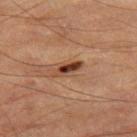Impression: Recorded during total-body skin imaging; not selected for excision or biopsy. Context: The recorded lesion diameter is about 3.5 mm. A male patient, in their mid-80s or older. A roughly 15 mm field-of-view crop from a total-body skin photograph. The lesion is on the leg. The tile uses cross-polarized illumination.